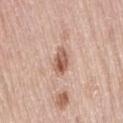Q: Was a biopsy performed?
A: total-body-photography surveillance lesion; no biopsy
Q: Automated lesion metrics?
A: a mean CIELAB color near L≈58 a*≈21 b*≈28, about 14 CIELAB-L* units darker than the surrounding skin, and a normalized border contrast of about 9; a border-irregularity index near 2.5/10, internal color variation of about 7.5 on a 0–10 scale, and a peripheral color-asymmetry measure near 3; a nevus-likeness score of about 95/100 and a detector confidence of about 100 out of 100 that the crop contains a lesion
Q: What are the patient's age and sex?
A: female, about 65 years old
Q: What is the imaging modality?
A: 15 mm crop, total-body photography
Q: What lighting was used for the tile?
A: white-light
Q: How large is the lesion?
A: ≈3.5 mm
Q: Lesion location?
A: the right thigh